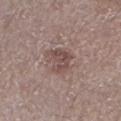Captured during whole-body skin photography for melanoma surveillance; the lesion was not biopsied.
A male patient, aged 63–67.
The lesion is on the left lower leg.
Imaged with white-light lighting.
A lesion tile, about 15 mm wide, cut from a 3D total-body photograph.